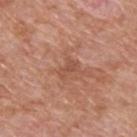Case summary:
– follow-up · no biopsy performed (imaged during a skin exam)
– diameter · ≈2.5 mm
– location · the back
– subject · male, roughly 60 years of age
– image source · ~15 mm crop, total-body skin-cancer survey
– image-analysis metrics · a lesion area of about 3.5 mm², an eccentricity of roughly 0.75, and two-axis asymmetry of about 0.3; a border-irregularity index near 3.5/10 and radial color variation of about 0
– lighting · white-light